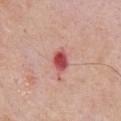notes=imaged on a skin check; not biopsied | size=≈3 mm | site=the chest | patient=male, aged around 65 | lighting=white-light | imaging modality=total-body-photography crop, ~15 mm field of view.